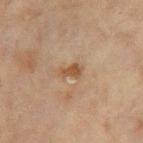notes: imaged on a skin check; not biopsied
lesion diameter: ~2.5 mm (longest diameter)
TBP lesion metrics: a lesion area of about 3.5 mm², an outline eccentricity of about 0.8 (0 = round, 1 = elongated), and a symmetry-axis asymmetry near 0.5; a border-irregularity rating of about 4.5/10, a within-lesion color-variation index near 1/10, and peripheral color asymmetry of about 0
tile lighting: cross-polarized
acquisition: ~15 mm tile from a whole-body skin photo
patient: female, about 80 years old
site: the right thigh